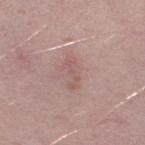notes = catalogued during a skin exam; not biopsied | lesion size = about 3.5 mm | subject = male, roughly 40 years of age | anatomic site = the right thigh | illumination = white-light illumination | image = ~15 mm crop, total-body skin-cancer survey.